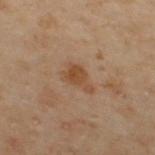biopsy_status: not biopsied; imaged during a skin examination
lighting: cross-polarized
image:
  source: total-body photography crop
  field_of_view_mm: 15
automated_metrics:
  area_mm2_approx: 6.0
  eccentricity: 0.8
  shape_asymmetry: 0.4
  cielab_L: 38
  cielab_a: 16
  cielab_b: 27
  vs_skin_darker_L: 7.0
  vs_skin_contrast_norm: 7.0
site: upper back
lesion_size:
  long_diameter_mm_approx: 3.5
patient:
  sex: male
  age_approx: 50